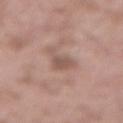| field | value |
|---|---|
| workup | total-body-photography surveillance lesion; no biopsy |
| image | ~15 mm crop, total-body skin-cancer survey |
| site | the lower back |
| lesion diameter | ≈3.5 mm |
| patient | male, aged 53 to 57 |
| automated metrics | about 9 CIELAB-L* units darker than the surrounding skin and a normalized border contrast of about 6.5; border irregularity of about 5.5 on a 0–10 scale; a nevus-likeness score of about 0/100 and lesion-presence confidence of about 100/100 |